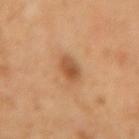notes: total-body-photography surveillance lesion; no biopsy
illumination: cross-polarized
image: ~15 mm tile from a whole-body skin photo
anatomic site: the left forearm
image-analysis metrics: an average lesion color of about L≈52 a*≈23 b*≈37 (CIELAB) and roughly 11 lightness units darker than nearby skin; border irregularity of about 1.5 on a 0–10 scale and a color-variation rating of about 3.5/10; an automated nevus-likeness rating near 85 out of 100 and lesion-presence confidence of about 100/100
subject: female, aged approximately 35